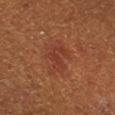Imaged during a routine full-body skin examination; the lesion was not biopsied and no histopathology is available.
Approximately 4.5 mm at its widest.
On the right lower leg.
The patient is a male in their mid-50s.
Captured under cross-polarized illumination.
A 15 mm crop from a total-body photograph taken for skin-cancer surveillance.